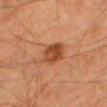size — about 3.5 mm | patient — male, aged around 80 | body site — the right thigh | imaging modality — total-body-photography crop, ~15 mm field of view.